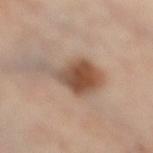Imaged during a routine full-body skin examination; the lesion was not biopsied and no histopathology is available.
Measured at roughly 7 mm in maximum diameter.
The lesion-visualizer software estimated a footprint of about 15 mm², an outline eccentricity of about 0.85 (0 = round, 1 = elongated), and a symmetry-axis asymmetry near 0.5. The software also gave a nevus-likeness score of about 95/100 and a detector confidence of about 100 out of 100 that the crop contains a lesion.
A 15 mm close-up tile from a total-body photography series done for melanoma screening.
From the leg.
A female patient, aged around 60.
Captured under cross-polarized illumination.